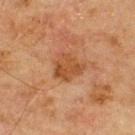biopsy status = imaged on a skin check; not biopsied | patient = male, in their 70s | anatomic site = the upper back | image = 15 mm crop, total-body photography.